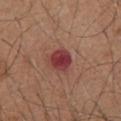Image and clinical context:
This image is a 15 mm lesion crop taken from a total-body photograph. The patient is a male in their mid-70s. Captured under white-light illumination. On the chest. Automated tile analysis of the lesion measured a footprint of about 6 mm² and a shape eccentricity near 0.3. And it measured a lesion-to-skin contrast of about 10.5 (normalized; higher = more distinct). The software also gave a classifier nevus-likeness of about 0/100 and a lesion-detection confidence of about 100/100. About 3 mm across.